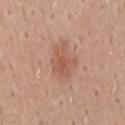follow-up: no biopsy performed (imaged during a skin exam); image: total-body-photography crop, ~15 mm field of view; patient: male, aged around 40; site: the back.